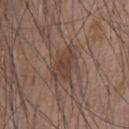Imaged during a routine full-body skin examination; the lesion was not biopsied and no histopathology is available. The patient is a male aged around 55. An algorithmic analysis of the crop reported an area of roughly 9.5 mm² and a shape-asymmetry score of about 0.25 (0 = symmetric). It also reported an average lesion color of about L≈41 a*≈17 b*≈24 (CIELAB) and a normalized border contrast of about 6.5. And it measured a nevus-likeness score of about 0/100. About 5 mm across. A 15 mm close-up extracted from a 3D total-body photography capture. The lesion is located on the chest.